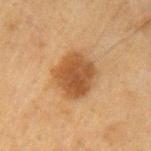Clinical impression: Imaged during a routine full-body skin examination; the lesion was not biopsied and no histopathology is available. Context: On the left upper arm. The lesion-visualizer software estimated an area of roughly 15 mm² and an eccentricity of roughly 0.5. It also reported about 11 CIELAB-L* units darker than the surrounding skin and a normalized lesion–skin contrast near 9. The software also gave lesion-presence confidence of about 100/100. The subject is a male aged around 50. A 15 mm crop from a total-body photograph taken for skin-cancer surveillance. Captured under cross-polarized illumination.